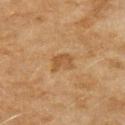No biopsy was performed on this lesion — it was imaged during a full skin examination and was not determined to be concerning.
The lesion is located on the left upper arm.
The tile uses cross-polarized illumination.
This image is a 15 mm lesion crop taken from a total-body photograph.
Automated tile analysis of the lesion measured a lesion color around L≈49 a*≈19 b*≈36 in CIELAB, roughly 8 lightness units darker than nearby skin, and a normalized border contrast of about 6. It also reported a peripheral color-asymmetry measure near 0.5. The analysis additionally found a nevus-likeness score of about 0/100 and a lesion-detection confidence of about 100/100.
A female patient, in their 60s.